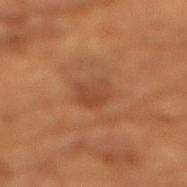| key | value |
|---|---|
| biopsy status | total-body-photography surveillance lesion; no biopsy |
| diameter | about 3.5 mm |
| automated metrics | a border-irregularity index near 2.5/10 and internal color variation of about 2.5 on a 0–10 scale |
| anatomic site | the chest |
| subject | female, in their 80s |
| tile lighting | cross-polarized illumination |
| acquisition | ~15 mm tile from a whole-body skin photo |